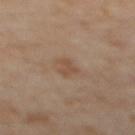The subject is a female aged around 60.
Imaged with cross-polarized lighting.
A region of skin cropped from a whole-body photographic capture, roughly 15 mm wide.
Automated image analysis of the tile measured an area of roughly 3.5 mm², an eccentricity of roughly 0.75, and a shape-asymmetry score of about 0.35 (0 = symmetric). The software also gave a classifier nevus-likeness of about 15/100 and lesion-presence confidence of about 100/100.
The lesion is on the upper back.